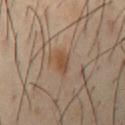{
  "biopsy_status": "not biopsied; imaged during a skin examination"
}